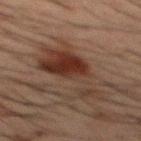{"biopsy_status": "not biopsied; imaged during a skin examination", "lighting": "cross-polarized", "image": {"source": "total-body photography crop", "field_of_view_mm": 15}, "automated_metrics": {"eccentricity": 0.9, "shape_asymmetry": 0.5, "vs_skin_darker_L": 8.0}, "site": "back", "lesion_size": {"long_diameter_mm_approx": 9.0}, "patient": {"sex": "male", "age_approx": 50}}